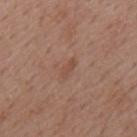workup=catalogued during a skin exam; not biopsied | subject=male, roughly 60 years of age | location=the mid back | imaging modality=total-body-photography crop, ~15 mm field of view.